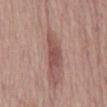{
  "biopsy_status": "not biopsied; imaged during a skin examination",
  "lighting": "white-light",
  "site": "front of the torso",
  "patient": {
    "sex": "male",
    "age_approx": 70
  },
  "lesion_size": {
    "long_diameter_mm_approx": 5.0
  },
  "image": {
    "source": "total-body photography crop",
    "field_of_view_mm": 15
  }
}